On the left lower leg, a roughly 15 mm field-of-view crop from a total-body skin photograph, the subject is a male in their 40s: 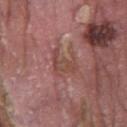Conclusion:
Histopathologically confirmed as a dysplastic (Clark) nevus.